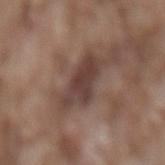Imaged during a routine full-body skin examination; the lesion was not biopsied and no histopathology is available. Located on the lower back. A male subject aged 73–77. The tile uses white-light illumination. A close-up tile cropped from a whole-body skin photograph, about 15 mm across.